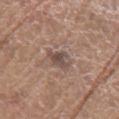| field | value |
|---|---|
| workup | catalogued during a skin exam; not biopsied |
| image | ~15 mm tile from a whole-body skin photo |
| body site | the left upper arm |
| patient | male, approximately 80 years of age |